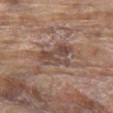Assessment:
Recorded during total-body skin imaging; not selected for excision or biopsy.
Background:
Longest diameter approximately 4.5 mm. Located on the chest. Automated tile analysis of the lesion measured a border-irregularity index near 5/10, a within-lesion color-variation index near 4/10, and radial color variation of about 1.5. The software also gave a detector confidence of about 60 out of 100 that the crop contains a lesion. A male subject, approximately 80 years of age. A region of skin cropped from a whole-body photographic capture, roughly 15 mm wide.